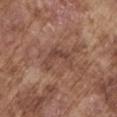Clinical impression: Captured during whole-body skin photography for melanoma surveillance; the lesion was not biopsied. Acquisition and patient details: A 15 mm crop from a total-body photograph taken for skin-cancer surveillance. The subject is a male about 75 years old. Captured under white-light illumination. Approximately 4 mm at its widest. On the right upper arm.